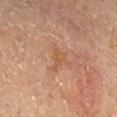Clinical impression: Recorded during total-body skin imaging; not selected for excision or biopsy. Image and clinical context: The lesion-visualizer software estimated a lesion area of about 3 mm², an eccentricity of roughly 0.9, and two-axis asymmetry of about 0.55. The analysis additionally found a mean CIELAB color near L≈43 a*≈18 b*≈28 and about 5 CIELAB-L* units darker than the surrounding skin. The software also gave a nevus-likeness score of about 0/100. A 15 mm close-up tile from a total-body photography series done for melanoma screening. The subject is a male in their mid- to late 60s. The tile uses cross-polarized illumination. Located on the right lower leg.